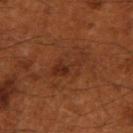{"biopsy_status": "not biopsied; imaged during a skin examination", "lighting": "cross-polarized", "patient": {"sex": "male", "age_approx": 50}, "site": "left arm", "automated_metrics": {"cielab_L": 30, "cielab_a": 24, "cielab_b": 31, "vs_skin_contrast_norm": 6.5, "border_irregularity_0_10": 8.5, "color_variation_0_10": 1.5}, "image": {"source": "total-body photography crop", "field_of_view_mm": 15}, "lesion_size": {"long_diameter_mm_approx": 4.5}}